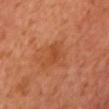The lesion was tiled from a total-body skin photograph and was not biopsied. Measured at roughly 3 mm in maximum diameter. Automated image analysis of the tile measured an area of roughly 4.5 mm², a shape eccentricity near 0.8, and a shape-asymmetry score of about 0.35 (0 = symmetric). The analysis additionally found a lesion color around L≈49 a*≈29 b*≈41 in CIELAB and a normalized border contrast of about 5.5. The analysis additionally found a border-irregularity index near 4/10 and a color-variation rating of about 2.5/10. And it measured a nevus-likeness score of about 0/100 and a lesion-detection confidence of about 100/100. Imaged with cross-polarized lighting. A female patient, aged around 55. From the chest. A close-up tile cropped from a whole-body skin photograph, about 15 mm across.